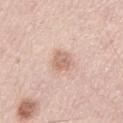Findings:
- workup: no biopsy performed (imaged during a skin exam)
- acquisition: 15 mm crop, total-body photography
- lesion diameter: about 2.5 mm
- illumination: white-light
- location: the left upper arm
- patient: male, aged 43 to 47